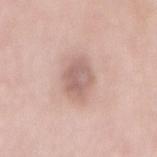workup=total-body-photography surveillance lesion; no biopsy
subject=female, aged 68–72
image=~15 mm crop, total-body skin-cancer survey
anatomic site=the mid back